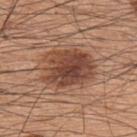The lesion was tiled from a total-body skin photograph and was not biopsied. On the upper back. Approximately 6.5 mm at its widest. Cropped from a total-body skin-imaging series; the visible field is about 15 mm. This is a white-light tile. A male subject aged approximately 60.